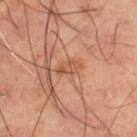notes: total-body-photography surveillance lesion; no biopsy | TBP lesion metrics: a mean CIELAB color near L≈51 a*≈24 b*≈33, a lesion–skin lightness drop of about 8, and a normalized border contrast of about 6; a border-irregularity index near 6/10 and peripheral color asymmetry of about 0; a nevus-likeness score of about 0/100 and a detector confidence of about 100 out of 100 that the crop contains a lesion | image: 15 mm crop, total-body photography | subject: male, aged approximately 60 | lighting: cross-polarized | location: the leg.